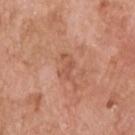No biopsy was performed on this lesion — it was imaged during a full skin examination and was not determined to be concerning. Located on the upper back. A close-up tile cropped from a whole-body skin photograph, about 15 mm across. This is a white-light tile. Automated image analysis of the tile measured an outline eccentricity of about 0.8 (0 = round, 1 = elongated) and a symmetry-axis asymmetry near 0.45. It also reported a lesion–skin lightness drop of about 7 and a normalized lesion–skin contrast near 5. It also reported an automated nevus-likeness rating near 0 out of 100 and lesion-presence confidence of about 100/100. Measured at roughly 3 mm in maximum diameter. The subject is a male about 60 years old.